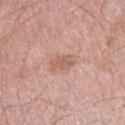Captured during whole-body skin photography for melanoma surveillance; the lesion was not biopsied.
The tile uses white-light illumination.
A roughly 15 mm field-of-view crop from a total-body skin photograph.
The lesion is on the right upper arm.
A male subject, in their 50s.